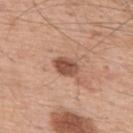The lesion was tiled from a total-body skin photograph and was not biopsied. The subject is a male aged around 55. On the upper back. The lesion-visualizer software estimated a lesion color around L≈50 a*≈23 b*≈29 in CIELAB, roughly 14 lightness units darker than nearby skin, and a lesion-to-skin contrast of about 9.5 (normalized; higher = more distinct). Cropped from a total-body skin-imaging series; the visible field is about 15 mm.